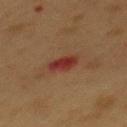Case summary:
* follow-up: total-body-photography surveillance lesion; no biopsy
* lesion diameter: about 3 mm
* illumination: cross-polarized
* patient: male, aged 53 to 57
* image: ~15 mm crop, total-body skin-cancer survey
* location: the mid back